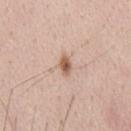The lesion was tiled from a total-body skin photograph and was not biopsied. A male subject aged approximately 45. From the chest. A roughly 15 mm field-of-view crop from a total-body skin photograph.